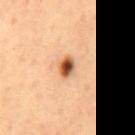Notes:
* follow-up — total-body-photography surveillance lesion; no biopsy
* image-analysis metrics — an area of roughly 4 mm², a shape eccentricity near 0.7, and two-axis asymmetry of about 0.15; a border-irregularity index near 1/10, a color-variation rating of about 9.5/10, and a peripheral color-asymmetry measure near 3; a nevus-likeness score of about 100/100 and lesion-presence confidence of about 100/100
* illumination — cross-polarized
* patient — male, in their mid- to late 50s
* lesion diameter — ~2.5 mm (longest diameter)
* site — the mid back
* image source — 15 mm crop, total-body photography